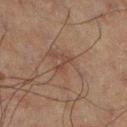  biopsy_status: not biopsied; imaged during a skin examination
  automated_metrics:
    area_mm2_approx: 2.5
    eccentricity: 0.9
    shape_asymmetry: 0.5
    nevus_likeness_0_100: 0
  patient:
    sex: male
    age_approx: 60
  lighting: cross-polarized
  site: left leg
  image:
    source: total-body photography crop
    field_of_view_mm: 15